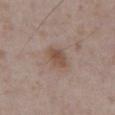Captured during whole-body skin photography for melanoma surveillance; the lesion was not biopsied.